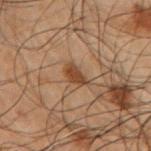<case>
  <biopsy_status>not biopsied; imaged during a skin examination</biopsy_status>
  <patient>
    <sex>male</sex>
    <age_approx>50</age_approx>
  </patient>
  <site>right upper arm</site>
  <lighting>cross-polarized</lighting>
  <image>
    <source>total-body photography crop</source>
    <field_of_view_mm>15</field_of_view_mm>
  </image>
  <lesion_size>
    <long_diameter_mm_approx>2.5</long_diameter_mm_approx>
  </lesion_size>
</case>